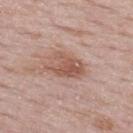Impression: This lesion was catalogued during total-body skin photography and was not selected for biopsy. Background: Approximately 5 mm at its widest. A region of skin cropped from a whole-body photographic capture, roughly 15 mm wide. The tile uses white-light illumination. The patient is a female approximately 50 years of age. Automated image analysis of the tile measured border irregularity of about 4.5 on a 0–10 scale, a color-variation rating of about 4/10, and a peripheral color-asymmetry measure near 1.5. From the upper back.